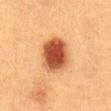Impression:
The lesion was photographed on a routine skin check and not biopsied; there is no pathology result.
Image and clinical context:
The lesion is located on the abdomen. A 15 mm close-up tile from a total-body photography series done for melanoma screening. Imaged with cross-polarized lighting. The total-body-photography lesion software estimated a lesion color around L≈44 a*≈25 b*≈33 in CIELAB, a lesion–skin lightness drop of about 16, and a normalized border contrast of about 12. The subject is a female aged around 40. The lesion's longest dimension is about 5 mm.